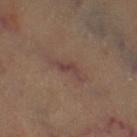Clinical impression: This lesion was catalogued during total-body skin photography and was not selected for biopsy. Context: Automated image analysis of the tile measured a footprint of about 3.5 mm², a shape eccentricity near 0.9, and a symmetry-axis asymmetry near 0.5. The analysis additionally found roughly 7 lightness units darker than nearby skin and a normalized lesion–skin contrast near 6.5. On the left thigh. A 15 mm close-up extracted from a 3D total-body photography capture. About 3.5 mm across. Captured under cross-polarized illumination. A subject roughly 60 years of age.